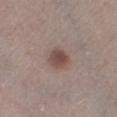{"biopsy_status": "not biopsied; imaged during a skin examination", "site": "right lower leg", "patient": {"sex": "male", "age_approx": 60}, "lesion_size": {"long_diameter_mm_approx": 2.5}, "image": {"source": "total-body photography crop", "field_of_view_mm": 15}}